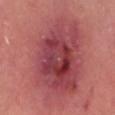workup = imaged on a skin check; not biopsied | diameter = ~17.5 mm (longest diameter) | subject = male, approximately 65 years of age | body site = the head or neck | acquisition = 15 mm crop, total-body photography | tile lighting = white-light.